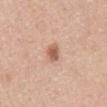Clinical impression: Recorded during total-body skin imaging; not selected for excision or biopsy.